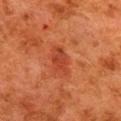| field | value |
|---|---|
| biopsy status | imaged on a skin check; not biopsied |
| image | ~15 mm tile from a whole-body skin photo |
| image-analysis metrics | a footprint of about 6 mm², an outline eccentricity of about 0.8 (0 = round, 1 = elongated), and a symmetry-axis asymmetry near 0.3; a lesion color around L≈36 a*≈30 b*≈33 in CIELAB, about 7 CIELAB-L* units darker than the surrounding skin, and a normalized border contrast of about 6; a nevus-likeness score of about 15/100 and a detector confidence of about 100 out of 100 that the crop contains a lesion |
| tile lighting | cross-polarized illumination |
| location | the back |
| diameter | ~3.5 mm (longest diameter) |
| subject | female, aged 48 to 52 |